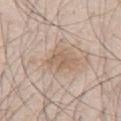biopsy_status: not biopsied; imaged during a skin examination
image:
  source: total-body photography crop
  field_of_view_mm: 15
site: abdomen
patient:
  sex: male
  age_approx: 45
lighting: white-light